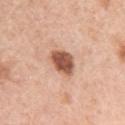Notes:
* workup — total-body-photography surveillance lesion; no biopsy
* lesion size — ~3.5 mm (longest diameter)
* imaging modality — ~15 mm tile from a whole-body skin photo
* illumination — white-light illumination
* body site — the left upper arm
* subject — female, aged 58–62